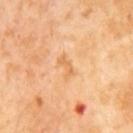No biopsy was performed on this lesion — it was imaged during a full skin examination and was not determined to be concerning. A lesion tile, about 15 mm wide, cut from a 3D total-body photograph. About 3 mm across. A male subject, aged 63–67. Imaged with cross-polarized lighting.